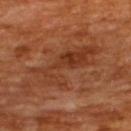Notes:
– notes · imaged on a skin check; not biopsied
– patient · male, in their mid- to late 60s
– image source · total-body-photography crop, ~15 mm field of view
– lesion diameter · ~8 mm (longest diameter)
– location · the upper back
– TBP lesion metrics · about 7 CIELAB-L* units darker than the surrounding skin
– tile lighting · cross-polarized illumination